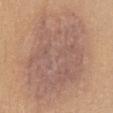illumination = white-light illumination | image = ~15 mm tile from a whole-body skin photo | size = about 13 mm | patient = female, aged around 25 | site = the abdomen.